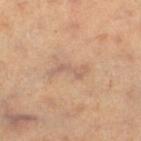Q: Is there a histopathology result?
A: catalogued during a skin exam; not biopsied
Q: Lesion location?
A: the leg
Q: What did automated image analysis measure?
A: a footprint of about 4 mm²; a mean CIELAB color near L≈59 a*≈18 b*≈28 and a lesion-to-skin contrast of about 5 (normalized; higher = more distinct); a border-irregularity rating of about 8/10 and a within-lesion color-variation index near 0/10; a nevus-likeness score of about 0/100 and lesion-presence confidence of about 95/100
Q: Illumination type?
A: cross-polarized illumination
Q: Who is the patient?
A: female, aged 48 to 52
Q: What is the imaging modality?
A: ~15 mm crop, total-body skin-cancer survey